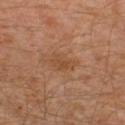biopsy_status: not biopsied; imaged during a skin examination
image:
  source: total-body photography crop
  field_of_view_mm: 15
lesion_size:
  long_diameter_mm_approx: 3.0
patient:
  sex: male
  age_approx: 30
lighting: cross-polarized
site: leg